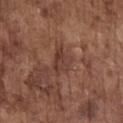Assessment:
Recorded during total-body skin imaging; not selected for excision or biopsy.
Acquisition and patient details:
On the chest. The recorded lesion diameter is about 3.5 mm. A male subject, about 75 years old. The tile uses white-light illumination. This image is a 15 mm lesion crop taken from a total-body photograph. The total-body-photography lesion software estimated an outline eccentricity of about 0.75 (0 = round, 1 = elongated). The software also gave a mean CIELAB color near L≈38 a*≈20 b*≈24, a lesion–skin lightness drop of about 8, and a normalized border contrast of about 7. The software also gave a peripheral color-asymmetry measure near 1.5. And it measured an automated nevus-likeness rating near 0 out of 100.